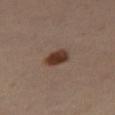Imaged during a routine full-body skin examination; the lesion was not biopsied and no histopathology is available.
An algorithmic analysis of the crop reported an average lesion color of about L≈36 a*≈18 b*≈25 (CIELAB), about 13 CIELAB-L* units darker than the surrounding skin, and a lesion-to-skin contrast of about 11.5 (normalized; higher = more distinct). The software also gave a classifier nevus-likeness of about 100/100.
The tile uses cross-polarized illumination.
A female patient, aged approximately 40.
From the right thigh.
A close-up tile cropped from a whole-body skin photograph, about 15 mm across.